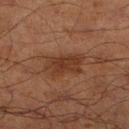Imaged during a routine full-body skin examination; the lesion was not biopsied and no histopathology is available.